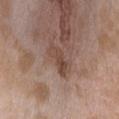Clinical summary:
A female subject, in their mid- to late 20s. Imaged with white-light lighting. The recorded lesion diameter is about 4 mm. A 15 mm close-up tile from a total-body photography series done for melanoma screening. The lesion is on the head or neck.